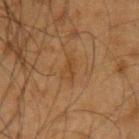workup: catalogued during a skin exam; not biopsied | subject: male, roughly 65 years of age | body site: the left upper arm | size: ~3 mm (longest diameter) | imaging modality: ~15 mm tile from a whole-body skin photo | tile lighting: cross-polarized.